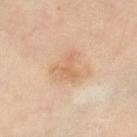This lesion was catalogued during total-body skin photography and was not selected for biopsy. A lesion tile, about 15 mm wide, cut from a 3D total-body photograph. On the right thigh. This is a cross-polarized tile. Automated image analysis of the tile measured an area of roughly 5 mm² and two-axis asymmetry of about 0.65. The software also gave an average lesion color of about L≈64 a*≈19 b*≈35 (CIELAB) and roughly 7 lightness units darker than nearby skin. The subject is a female aged around 60. The recorded lesion diameter is about 3.5 mm.